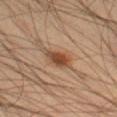The lesion was photographed on a routine skin check and not biopsied; there is no pathology result.